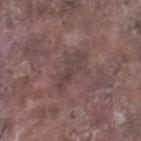The lesion was tiled from a total-body skin photograph and was not biopsied. The subject is a male aged 73 to 77. On the right lower leg. The tile uses white-light illumination. A lesion tile, about 15 mm wide, cut from a 3D total-body photograph. Measured at roughly 4.5 mm in maximum diameter.